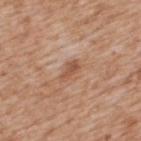Clinical impression:
Captured during whole-body skin photography for melanoma surveillance; the lesion was not biopsied.
Context:
The subject is a male aged 63 to 67. The lesion's longest dimension is about 3 mm. The lesion-visualizer software estimated a footprint of about 3 mm² and an eccentricity of roughly 0.9. The analysis additionally found roughly 9 lightness units darker than nearby skin. And it measured a border-irregularity index near 3.5/10, a within-lesion color-variation index near 0.5/10, and peripheral color asymmetry of about 0. And it measured a nevus-likeness score of about 0/100 and lesion-presence confidence of about 100/100. Captured under white-light illumination. A 15 mm crop from a total-body photograph taken for skin-cancer surveillance. On the upper back.